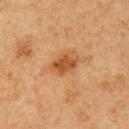Assessment:
Part of a total-body skin-imaging series; this lesion was reviewed on a skin check and was not flagged for biopsy.
Context:
The subject is a male aged around 60. The tile uses cross-polarized illumination. From the left upper arm. A lesion tile, about 15 mm wide, cut from a 3D total-body photograph. The recorded lesion diameter is about 3 mm.